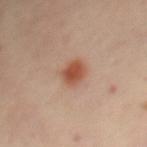Clinical impression: No biopsy was performed on this lesion — it was imaged during a full skin examination and was not determined to be concerning. Context: A lesion tile, about 15 mm wide, cut from a 3D total-body photograph. This is a cross-polarized tile. A female patient in their mid- to late 40s. From the chest. Automated tile analysis of the lesion measured an outline eccentricity of about 0.5 (0 = round, 1 = elongated) and a shape-asymmetry score of about 0.15 (0 = symmetric). The software also gave a mean CIELAB color near L≈51 a*≈24 b*≈31 and a lesion-to-skin contrast of about 9 (normalized; higher = more distinct). And it measured a color-variation rating of about 2.5/10 and peripheral color asymmetry of about 0.5. It also reported a nevus-likeness score of about 100/100 and a lesion-detection confidence of about 100/100. The lesion's longest dimension is about 3 mm.